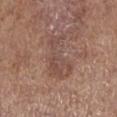biopsy_status: not biopsied; imaged during a skin examination
lighting: white-light
site: right lower leg
image:
  source: total-body photography crop
  field_of_view_mm: 15
patient:
  sex: male
  age_approx: 75
automated_metrics:
  cielab_L: 48
  cielab_a: 18
  cielab_b: 24
  vs_skin_darker_L: 7.0
  vs_skin_contrast_norm: 5.0
  color_variation_0_10: 3.5